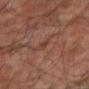The lesion was tiled from a total-body skin photograph and was not biopsied.
The lesion is located on the right forearm.
Imaged with cross-polarized lighting.
A male patient about 65 years old.
Cropped from a total-body skin-imaging series; the visible field is about 15 mm.